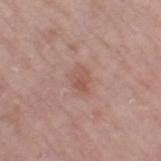| field | value |
|---|---|
| subject | female, in their mid-60s |
| automated lesion analysis | an area of roughly 5.5 mm², a shape eccentricity near 0.75, and two-axis asymmetry of about 0.25; an average lesion color of about L≈55 a*≈21 b*≈25 (CIELAB), a lesion–skin lightness drop of about 7, and a lesion-to-skin contrast of about 5.5 (normalized; higher = more distinct); a border-irregularity index near 2.5/10 and a peripheral color-asymmetry measure near 1.5; an automated nevus-likeness rating near 5 out of 100 and a lesion-detection confidence of about 100/100 |
| imaging modality | 15 mm crop, total-body photography |
| body site | the right thigh |